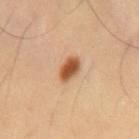Clinical impression: Part of a total-body skin-imaging series; this lesion was reviewed on a skin check and was not flagged for biopsy. Background: About 3 mm across. Automated tile analysis of the lesion measured a mean CIELAB color near L≈52 a*≈23 b*≈36 and about 16 CIELAB-L* units darker than the surrounding skin. This is a cross-polarized tile. This image is a 15 mm lesion crop taken from a total-body photograph. Located on the mid back. A male subject aged 53–57.